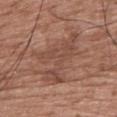Clinical impression:
Recorded during total-body skin imaging; not selected for excision or biopsy.
Background:
This is a white-light tile. The lesion's longest dimension is about 6.5 mm. A roughly 15 mm field-of-view crop from a total-body skin photograph. On the upper back. The subject is a male approximately 50 years of age.